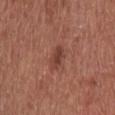Q: Is there a histopathology result?
A: catalogued during a skin exam; not biopsied
Q: Where on the body is the lesion?
A: the chest
Q: What is the imaging modality?
A: ~15 mm crop, total-body skin-cancer survey
Q: Illumination type?
A: white-light
Q: How large is the lesion?
A: ≈3 mm
Q: What are the patient's age and sex?
A: male, about 75 years old